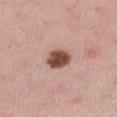Impression:
This lesion was catalogued during total-body skin photography and was not selected for biopsy.
Clinical summary:
Cropped from a whole-body photographic skin survey; the tile spans about 15 mm. The tile uses white-light illumination. Automated tile analysis of the lesion measured a footprint of about 7.5 mm², an outline eccentricity of about 0.6 (0 = round, 1 = elongated), and two-axis asymmetry of about 0.1. The software also gave a border-irregularity index near 1/10 and internal color variation of about 4 on a 0–10 scale. A female patient, approximately 35 years of age. On the right thigh.